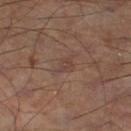Notes:
- follow-up — imaged on a skin check; not biopsied
- illumination — cross-polarized illumination
- patient — male, roughly 55 years of age
- lesion diameter — ≈3 mm
- body site — the left lower leg
- image — ~15 mm crop, total-body skin-cancer survey